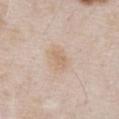This lesion was catalogued during total-body skin photography and was not selected for biopsy.
Approximately 3 mm at its widest.
An algorithmic analysis of the crop reported an area of roughly 5 mm² and a symmetry-axis asymmetry near 0.2. It also reported a nevus-likeness score of about 10/100 and a detector confidence of about 100 out of 100 that the crop contains a lesion.
Located on the chest.
Imaged with white-light lighting.
This image is a 15 mm lesion crop taken from a total-body photograph.
The subject is a male aged around 55.